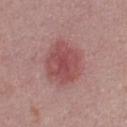Assessment: This lesion was catalogued during total-body skin photography and was not selected for biopsy. Background: A region of skin cropped from a whole-body photographic capture, roughly 15 mm wide. Captured under white-light illumination. The recorded lesion diameter is about 5 mm. A male subject aged 28 to 32.